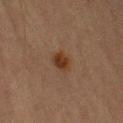Assessment: Recorded during total-body skin imaging; not selected for excision or biopsy. Clinical summary: This is a cross-polarized tile. A male patient, aged 63 to 67. The lesion-visualizer software estimated a footprint of about 4 mm², an eccentricity of roughly 0.7, and a symmetry-axis asymmetry near 0.2. It also reported roughly 8 lightness units darker than nearby skin and a normalized lesion–skin contrast near 9.5. The software also gave a color-variation rating of about 1.5/10. And it measured lesion-presence confidence of about 100/100. Cropped from a whole-body photographic skin survey; the tile spans about 15 mm. Approximately 2.5 mm at its widest. The lesion is located on the right upper arm.